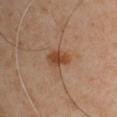Recorded during total-body skin imaging; not selected for excision or biopsy. About 3.5 mm across. A 15 mm crop from a total-body photograph taken for skin-cancer surveillance. An algorithmic analysis of the crop reported a border-irregularity rating of about 2/10 and a within-lesion color-variation index near 3/10. On the left upper arm. This is a cross-polarized tile. The subject is a male in their mid-40s.